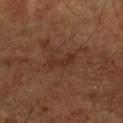Impression:
Imaged during a routine full-body skin examination; the lesion was not biopsied and no histopathology is available.
Background:
A patient roughly 65 years of age. A region of skin cropped from a whole-body photographic capture, roughly 15 mm wide. Located on the left lower leg.